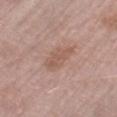Notes:
• notes · imaged on a skin check; not biopsied
• size · about 4.5 mm
• anatomic site · the right thigh
• subject · male, approximately 80 years of age
• lighting · white-light illumination
• imaging modality · 15 mm crop, total-body photography
• automated metrics · a border-irregularity index near 3/10, a color-variation rating of about 2/10, and radial color variation of about 0.5; a nevus-likeness score of about 5/100 and a detector confidence of about 100 out of 100 that the crop contains a lesion